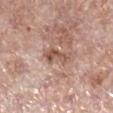<tbp_lesion>
  <biopsy_status>not biopsied; imaged during a skin examination</biopsy_status>
  <site>right lower leg</site>
  <patient>
    <sex>female</sex>
    <age_approx>70</age_approx>
  </patient>
  <lesion_size>
    <long_diameter_mm_approx>4.0</long_diameter_mm_approx>
  </lesion_size>
  <image>
    <source>total-body photography crop</source>
    <field_of_view_mm>15</field_of_view_mm>
  </image>
</tbp_lesion>